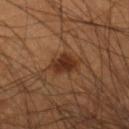Assessment:
The lesion was tiled from a total-body skin photograph and was not biopsied.
Clinical summary:
An algorithmic analysis of the crop reported a lesion area of about 7.5 mm², an eccentricity of roughly 0.8, and two-axis asymmetry of about 0.25. Measured at roughly 4 mm in maximum diameter. The tile uses cross-polarized illumination. Located on the right lower leg. The subject is a male aged 43 to 47. A lesion tile, about 15 mm wide, cut from a 3D total-body photograph.